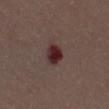Captured during whole-body skin photography for melanoma surveillance; the lesion was not biopsied.